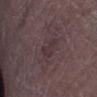This lesion was catalogued during total-body skin photography and was not selected for biopsy.
Captured under white-light illumination.
A male patient, in their mid-70s.
Located on the right lower leg.
Longest diameter approximately 3 mm.
This image is a 15 mm lesion crop taken from a total-body photograph.
Automated tile analysis of the lesion measured a classifier nevus-likeness of about 0/100.